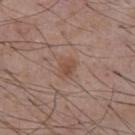The lesion was tiled from a total-body skin photograph and was not biopsied. Longest diameter approximately 2.5 mm. Cropped from a whole-body photographic skin survey; the tile spans about 15 mm. This is a white-light tile. From the chest. The patient is a male aged 53–57.